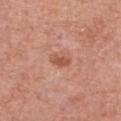{
  "biopsy_status": "not biopsied; imaged during a skin examination",
  "image": {
    "source": "total-body photography crop",
    "field_of_view_mm": 15
  },
  "patient": {
    "sex": "female",
    "age_approx": 75
  },
  "site": "chest"
}